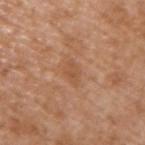Notes:
• follow-up — catalogued during a skin exam; not biopsied
• body site — the right upper arm
• acquisition — 15 mm crop, total-body photography
• tile lighting — white-light
• lesion size — about 3 mm
• automated metrics — a lesion area of about 4 mm² and a shape-asymmetry score of about 0.4 (0 = symmetric); an average lesion color of about L≈53 a*≈22 b*≈35 (CIELAB), a lesion–skin lightness drop of about 7, and a lesion-to-skin contrast of about 5 (normalized; higher = more distinct); a border-irregularity rating of about 3.5/10 and radial color variation of about 0.5; a detector confidence of about 100 out of 100 that the crop contains a lesion
• subject — male, about 65 years old